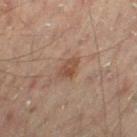{
  "biopsy_status": "not biopsied; imaged during a skin examination",
  "image": {
    "source": "total-body photography crop",
    "field_of_view_mm": 15
  },
  "site": "leg",
  "patient": {
    "sex": "male",
    "age_approx": 45
  },
  "lighting": "cross-polarized",
  "lesion_size": {
    "long_diameter_mm_approx": 3.0
  }
}